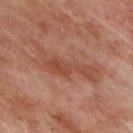biopsy_status: not biopsied; imaged during a skin examination
image:
  source: total-body photography crop
  field_of_view_mm: 15
lesion_size:
  long_diameter_mm_approx: 8.0
automated_metrics:
  eccentricity: 0.95
  shape_asymmetry: 0.45
  cielab_L: 47
  cielab_a: 23
  cielab_b: 30
  vs_skin_darker_L: 7.0
  border_irregularity_0_10: 7.5
  color_variation_0_10: 3.5
  peripheral_color_asymmetry: 1.0
patient:
  sex: male
  age_approx: 70
lighting: cross-polarized
site: upper back